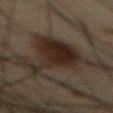{
  "biopsy_status": "not biopsied; imaged during a skin examination",
  "patient": {
    "sex": "male",
    "age_approx": 60
  },
  "lighting": "cross-polarized",
  "image": {
    "source": "total-body photography crop",
    "field_of_view_mm": 15
  },
  "automated_metrics": {
    "area_mm2_approx": 30.0,
    "eccentricity": 0.75,
    "shape_asymmetry": 0.65,
    "cielab_L": 19,
    "cielab_a": 9,
    "cielab_b": 16,
    "vs_skin_contrast_norm": 10.0,
    "border_irregularity_0_10": 9.5
  },
  "lesion_size": {
    "long_diameter_mm_approx": 9.5
  },
  "site": "chest"
}